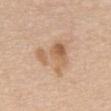| feature | finding |
|---|---|
| follow-up | catalogued during a skin exam; not biopsied |
| subject | female, aged 68–72 |
| imaging modality | ~15 mm tile from a whole-body skin photo |
| illumination | white-light |
| lesion diameter | ≈5.5 mm |
| anatomic site | the chest |
| TBP lesion metrics | an area of roughly 13 mm², a shape eccentricity near 0.5, and a symmetry-axis asymmetry near 0.6; a border-irregularity index near 8/10 and radial color variation of about 2.5; an automated nevus-likeness rating near 15 out of 100 and a lesion-detection confidence of about 100/100 |